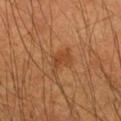Assessment:
Imaged during a routine full-body skin examination; the lesion was not biopsied and no histopathology is available.
Image and clinical context:
The lesion is located on the left upper arm. Approximately 3.5 mm at its widest. A lesion tile, about 15 mm wide, cut from a 3D total-body photograph. A male subject, aged 53 to 57.